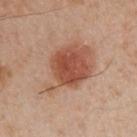The lesion's longest dimension is about 3.5 mm. A male patient, aged 48–52. Captured under cross-polarized illumination. Automated image analysis of the tile measured an automated nevus-likeness rating near 100 out of 100 and lesion-presence confidence of about 100/100. The lesion is located on the left arm. A close-up tile cropped from a whole-body skin photograph, about 15 mm across.